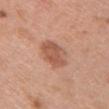Imaged during a routine full-body skin examination; the lesion was not biopsied and no histopathology is available. A close-up tile cropped from a whole-body skin photograph, about 15 mm across. A female subject about 60 years old. Captured under white-light illumination. The lesion is located on the chest.